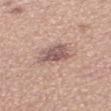Background: Located on the left thigh. This is a white-light tile. The recorded lesion diameter is about 5 mm. A female patient, in their 30s. A roughly 15 mm field-of-view crop from a total-body skin photograph. Automated tile analysis of the lesion measured an average lesion color of about L≈60 a*≈17 b*≈23 (CIELAB), roughly 8 lightness units darker than nearby skin, and a lesion-to-skin contrast of about 5.5 (normalized; higher = more distinct). The software also gave a within-lesion color-variation index near 6/10.